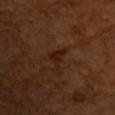Findings:
- follow-up · catalogued during a skin exam; not biopsied
- site · the upper back
- patient · female, aged 53–57
- illumination · cross-polarized
- automated metrics · an outline eccentricity of about 0.8 (0 = round, 1 = elongated) and a shape-asymmetry score of about 0.5 (0 = symmetric); an average lesion color of about L≈20 a*≈19 b*≈25 (CIELAB) and a lesion–skin lightness drop of about 7; a classifier nevus-likeness of about 0/100 and a lesion-detection confidence of about 100/100
- acquisition · total-body-photography crop, ~15 mm field of view
- size · ≈3 mm